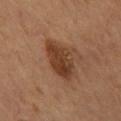{"biopsy_status": "not biopsied; imaged during a skin examination", "site": "mid back", "patient": {"sex": "male", "age_approx": 55}, "image": {"source": "total-body photography crop", "field_of_view_mm": 15}}